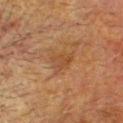Q: Was this lesion biopsied?
A: imaged on a skin check; not biopsied
Q: What are the patient's age and sex?
A: male, in their mid- to late 70s
Q: How was the tile lit?
A: cross-polarized illumination
Q: How was this image acquired?
A: ~15 mm tile from a whole-body skin photo
Q: Lesion size?
A: ≈3 mm
Q: Automated lesion metrics?
A: an area of roughly 4.5 mm², a shape eccentricity near 0.7, and a shape-asymmetry score of about 0.35 (0 = symmetric)
Q: Lesion location?
A: the head or neck